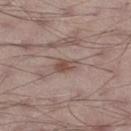Impression:
Imaged during a routine full-body skin examination; the lesion was not biopsied and no histopathology is available.
Context:
Captured under white-light illumination. The subject is a male about 70 years old. An algorithmic analysis of the crop reported an area of roughly 4 mm², an eccentricity of roughly 0.85, and two-axis asymmetry of about 0.3. On the left thigh. This image is a 15 mm lesion crop taken from a total-body photograph.